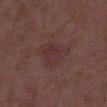Assessment:
This lesion was catalogued during total-body skin photography and was not selected for biopsy.
Image and clinical context:
A region of skin cropped from a whole-body photographic capture, roughly 15 mm wide. The subject is a male approximately 50 years of age. Automated tile analysis of the lesion measured a border-irregularity rating of about 3.5/10, a within-lesion color-variation index near 3/10, and radial color variation of about 1. This is a white-light tile.